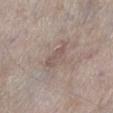Q: What kind of image is this?
A: ~15 mm tile from a whole-body skin photo
Q: Automated lesion metrics?
A: a footprint of about 4.5 mm² and two-axis asymmetry of about 0.3; a classifier nevus-likeness of about 0/100 and a lesion-detection confidence of about 65/100
Q: What are the patient's age and sex?
A: male, aged 58 to 62
Q: What is the lesion's diameter?
A: about 4 mm
Q: Where on the body is the lesion?
A: the left lower leg
Q: Illumination type?
A: white-light